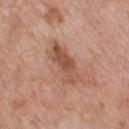This lesion was catalogued during total-body skin photography and was not selected for biopsy. The lesion is on the chest. The subject is a male aged around 60. Cropped from a total-body skin-imaging series; the visible field is about 15 mm.